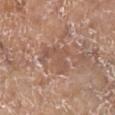Assessment: Captured during whole-body skin photography for melanoma surveillance; the lesion was not biopsied. Context: Cropped from a whole-body photographic skin survey; the tile spans about 15 mm. The tile uses white-light illumination. The subject is a female aged 73–77. The lesion is on the right lower leg.